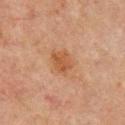Assessment:
The lesion was photographed on a routine skin check and not biopsied; there is no pathology result.
Background:
Captured under cross-polarized illumination. The lesion is on the upper back. The subject is a male in their mid-60s. About 3 mm across. A 15 mm close-up extracted from a 3D total-body photography capture.